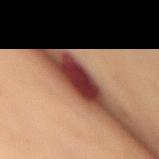| key | value |
|---|---|
| biopsy status | no biopsy performed (imaged during a skin exam) |
| lesion diameter | ≈5.5 mm |
| image | total-body-photography crop, ~15 mm field of view |
| patient | female, aged approximately 60 |
| image-analysis metrics | a lesion area of about 10 mm² and a symmetry-axis asymmetry near 0.15; a mean CIELAB color near L≈31 a*≈27 b*≈22; a border-irregularity rating of about 2.5/10, a within-lesion color-variation index near 3/10, and peripheral color asymmetry of about 1 |
| site | the front of the torso |
| illumination | cross-polarized illumination |